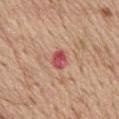  biopsy_status: not biopsied; imaged during a skin examination
  lighting: white-light
  site: mid back
  patient:
    sex: male
    age_approx: 70
  image:
    source: total-body photography crop
    field_of_view_mm: 15
  automated_metrics:
    vs_skin_darker_L: 12.0
    vs_skin_contrast_norm: 9.5
    border_irregularity_0_10: 2.5
    color_variation_0_10: 4.5
    nevus_likeness_0_100: 0
  lesion_size:
    long_diameter_mm_approx: 2.5